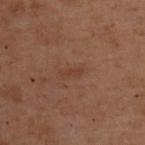{
  "biopsy_status": "not biopsied; imaged during a skin examination",
  "image": {
    "source": "total-body photography crop",
    "field_of_view_mm": 15
  },
  "lighting": "cross-polarized",
  "site": "upper back",
  "lesion_size": {
    "long_diameter_mm_approx": 2.5
  },
  "patient": {
    "sex": "female",
    "age_approx": 55
  }
}